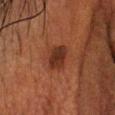Recorded during total-body skin imaging; not selected for excision or biopsy.
A male subject, approximately 60 years of age.
From the head or neck.
A 15 mm close-up tile from a total-body photography series done for melanoma screening.